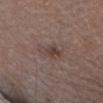Captured during whole-body skin photography for melanoma surveillance; the lesion was not biopsied. The subject is a female approximately 30 years of age. About 3.5 mm across. A 15 mm crop from a total-body photograph taken for skin-cancer surveillance. Located on the head or neck. Automated tile analysis of the lesion measured an area of roughly 4.5 mm², an eccentricity of roughly 0.85, and a symmetry-axis asymmetry near 0.35. It also reported a lesion color around L≈41 a*≈15 b*≈20 in CIELAB and a lesion-to-skin contrast of about 6.5 (normalized; higher = more distinct). The analysis additionally found internal color variation of about 2.5 on a 0–10 scale and a peripheral color-asymmetry measure near 1. The software also gave an automated nevus-likeness rating near 5 out of 100 and a lesion-detection confidence of about 100/100. This is a white-light tile.